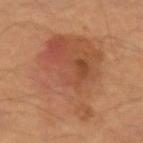Imaged during a routine full-body skin examination; the lesion was not biopsied and no histopathology is available. The recorded lesion diameter is about 10 mm. The lesion is located on the left forearm. A 15 mm close-up extracted from a 3D total-body photography capture. A male subject in their mid-50s. The total-body-photography lesion software estimated a footprint of about 50 mm² and an eccentricity of roughly 0.65. And it measured a border-irregularity rating of about 4/10, a within-lesion color-variation index near 6/10, and a peripheral color-asymmetry measure near 2. The analysis additionally found a nevus-likeness score of about 30/100 and a detector confidence of about 100 out of 100 that the crop contains a lesion.